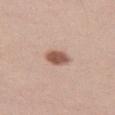Clinical impression: Part of a total-body skin-imaging series; this lesion was reviewed on a skin check and was not flagged for biopsy. Background: Imaged with white-light lighting. The lesion's longest dimension is about 3 mm. Cropped from a whole-body photographic skin survey; the tile spans about 15 mm. A female subject, aged 18–22. The lesion-visualizer software estimated a footprint of about 5.5 mm² and two-axis asymmetry of about 0.2. It also reported a color-variation rating of about 2/10 and peripheral color asymmetry of about 0.5. The software also gave an automated nevus-likeness rating near 100 out of 100. The lesion is located on the left thigh.